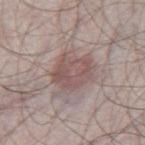Case summary:
- notes — imaged on a skin check; not biopsied
- patient — male, aged around 65
- diameter — ~4.5 mm (longest diameter)
- site — the right thigh
- tile lighting — white-light illumination
- acquisition — ~15 mm crop, total-body skin-cancer survey